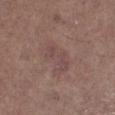The lesion was tiled from a total-body skin photograph and was not biopsied.
Measured at roughly 4 mm in maximum diameter.
A male patient aged 73–77.
Cropped from a whole-body photographic skin survey; the tile spans about 15 mm.
Located on the left lower leg.